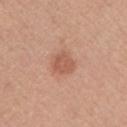Clinical summary:
From the front of the torso. Captured under white-light illumination. Cropped from a whole-body photographic skin survey; the tile spans about 15 mm. Longest diameter approximately 3 mm. A male subject, in their 30s. The total-body-photography lesion software estimated an eccentricity of roughly 0.5 and a symmetry-axis asymmetry near 0.2. The analysis additionally found a detector confidence of about 100 out of 100 that the crop contains a lesion.